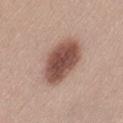biopsy status: total-body-photography surveillance lesion; no biopsy | location: the lower back | lesion diameter: about 6.5 mm | image source: 15 mm crop, total-body photography | illumination: white-light illumination | automated metrics: a lesion color around L≈51 a*≈20 b*≈25 in CIELAB and about 16 CIELAB-L* units darker than the surrounding skin; a border-irregularity rating of about 1.5/10 and a within-lesion color-variation index near 5/10; an automated nevus-likeness rating near 95 out of 100 and lesion-presence confidence of about 100/100 | subject: female, approximately 25 years of age.